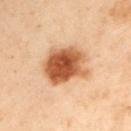The subject is a male aged approximately 55. The lesion's longest dimension is about 5.5 mm. A 15 mm close-up tile from a total-body photography series done for melanoma screening. Automated tile analysis of the lesion measured an average lesion color of about L≈46 a*≈21 b*≈33 (CIELAB) and roughly 16 lightness units darker than nearby skin. And it measured a border-irregularity rating of about 2.5/10, a within-lesion color-variation index near 6/10, and a peripheral color-asymmetry measure near 2. The lesion is located on the left upper arm.